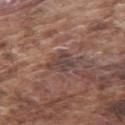biopsy status: no biopsy performed (imaged during a skin exam)
body site: the arm
illumination: white-light illumination
automated metrics: an eccentricity of roughly 0.55; a border-irregularity rating of about 3.5/10 and peripheral color asymmetry of about 1; an automated nevus-likeness rating near 0 out of 100 and a lesion-detection confidence of about 60/100
image: 15 mm crop, total-body photography
patient: male, approximately 75 years of age